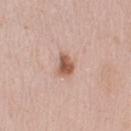{"biopsy_status": "not biopsied; imaged during a skin examination", "lesion_size": {"long_diameter_mm_approx": 2.5}, "patient": {"sex": "female", "age_approx": 40}, "lighting": "white-light", "site": "right upper arm", "image": {"source": "total-body photography crop", "field_of_view_mm": 15}}